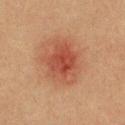Imaged during a routine full-body skin examination; the lesion was not biopsied and no histopathology is available.
A region of skin cropped from a whole-body photographic capture, roughly 15 mm wide.
Captured under cross-polarized illumination.
From the front of the torso.
Approximately 6 mm at its widest.
A male patient about 40 years old.